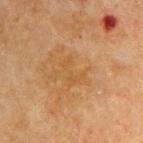Q: Was this lesion biopsied?
A: total-body-photography surveillance lesion; no biopsy
Q: What are the patient's age and sex?
A: male, about 70 years old
Q: Lesion size?
A: ≈3 mm
Q: What kind of image is this?
A: total-body-photography crop, ~15 mm field of view
Q: How was the tile lit?
A: cross-polarized illumination
Q: Where on the body is the lesion?
A: the upper back